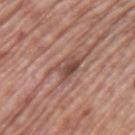This image is a 15 mm lesion crop taken from a total-body photograph.
From the left upper arm.
A male patient aged 68 to 72.